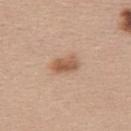  biopsy_status: not biopsied; imaged during a skin examination
  patient:
    sex: female
    age_approx: 40
  lesion_size:
    long_diameter_mm_approx: 3.5
  image:
    source: total-body photography crop
    field_of_view_mm: 15
  lighting: white-light
  site: upper back